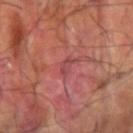  biopsy_status: not biopsied; imaged during a skin examination
  site: left forearm
  patient:
    sex: male
    age_approx: 60
  image:
    source: total-body photography crop
    field_of_view_mm: 15